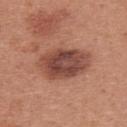This lesion was catalogued during total-body skin photography and was not selected for biopsy. The lesion is on the upper back. Automated image analysis of the tile measured an automated nevus-likeness rating near 50 out of 100 and a detector confidence of about 100 out of 100 that the crop contains a lesion. The subject is a female aged 38–42. A close-up tile cropped from a whole-body skin photograph, about 15 mm across.